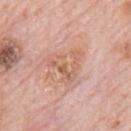{
  "biopsy_status": "not biopsied; imaged during a skin examination",
  "patient": {
    "sex": "male",
    "age_approx": 80
  },
  "lighting": "white-light",
  "site": "mid back",
  "lesion_size": {
    "long_diameter_mm_approx": 5.0
  },
  "image": {
    "source": "total-body photography crop",
    "field_of_view_mm": 15
  }
}